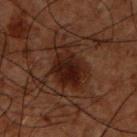Impression: Part of a total-body skin-imaging series; this lesion was reviewed on a skin check and was not flagged for biopsy. Image and clinical context: A region of skin cropped from a whole-body photographic capture, roughly 15 mm wide. The lesion is located on the back. A male patient aged 48–52. The lesion-visualizer software estimated a lesion area of about 14 mm², an eccentricity of roughly 0.8, and a shape-asymmetry score of about 0.3 (0 = symmetric). It also reported a lesion color around L≈15 a*≈16 b*≈17 in CIELAB, roughly 7 lightness units darker than nearby skin, and a lesion-to-skin contrast of about 10.5 (normalized; higher = more distinct). The analysis additionally found an automated nevus-likeness rating near 85 out of 100 and lesion-presence confidence of about 100/100. This is a cross-polarized tile. Approximately 6 mm at its widest.